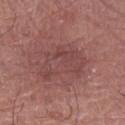Q: Was this lesion biopsied?
A: imaged on a skin check; not biopsied
Q: How was the tile lit?
A: white-light
Q: How was this image acquired?
A: total-body-photography crop, ~15 mm field of view
Q: Where on the body is the lesion?
A: the right forearm
Q: Automated lesion metrics?
A: a lesion color around L≈45 a*≈24 b*≈21 in CIELAB and about 6 CIELAB-L* units darker than the surrounding skin
Q: Who is the patient?
A: male, in their mid- to late 70s
Q: Lesion size?
A: ~6 mm (longest diameter)